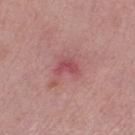  biopsy_status: not biopsied; imaged during a skin examination
  lighting: white-light
  image:
    source: total-body photography crop
    field_of_view_mm: 15
  site: leg
  patient:
    sex: female
    age_approx: 65